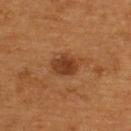Q: Was a biopsy performed?
A: no biopsy performed (imaged during a skin exam)
Q: Patient demographics?
A: male, roughly 55 years of age
Q: What is the imaging modality?
A: total-body-photography crop, ~15 mm field of view
Q: What is the lesion's diameter?
A: about 3 mm
Q: Lesion location?
A: the upper back